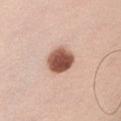The lesion was tiled from a total-body skin photograph and was not biopsied. The lesion is on the arm. This image is a 15 mm lesion crop taken from a total-body photograph. Approximately 3.5 mm at its widest. Captured under white-light illumination. A male patient approximately 35 years of age.